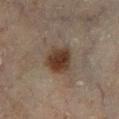Q: Was this lesion biopsied?
A: total-body-photography surveillance lesion; no biopsy
Q: How was the tile lit?
A: cross-polarized illumination
Q: Lesion size?
A: ~4 mm (longest diameter)
Q: Where on the body is the lesion?
A: the left lower leg
Q: How was this image acquired?
A: 15 mm crop, total-body photography
Q: What are the patient's age and sex?
A: male, aged approximately 70